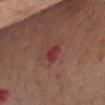{
  "biopsy_status": "not biopsied; imaged during a skin examination",
  "patient": {
    "sex": "female",
    "age_approx": 75
  },
  "site": "front of the torso",
  "automated_metrics": {
    "border_irregularity_0_10": 2.5,
    "color_variation_0_10": 4.0,
    "peripheral_color_asymmetry": 1.5
  },
  "image": {
    "source": "total-body photography crop",
    "field_of_view_mm": 15
  },
  "lesion_size": {
    "long_diameter_mm_approx": 2.5
  }
}